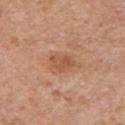Q: Was this lesion biopsied?
A: no biopsy performed (imaged during a skin exam)
Q: What are the patient's age and sex?
A: female, roughly 50 years of age
Q: Lesion location?
A: the right upper arm
Q: Automated lesion metrics?
A: a lesion area of about 4.5 mm² and a shape-asymmetry score of about 0.2 (0 = symmetric); a classifier nevus-likeness of about 20/100 and a lesion-detection confidence of about 100/100
Q: Lesion size?
A: about 2.5 mm
Q: What is the imaging modality?
A: ~15 mm crop, total-body skin-cancer survey